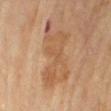Part of a total-body skin-imaging series; this lesion was reviewed on a skin check and was not flagged for biopsy.
From the left forearm.
Measured at roughly 8 mm in maximum diameter.
This is a cross-polarized tile.
Cropped from a total-body skin-imaging series; the visible field is about 15 mm.
A female subject, aged 78 to 82.